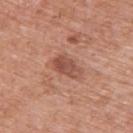Case summary:
- workup · total-body-photography surveillance lesion; no biopsy
- subject · male, aged 78–82
- anatomic site · the back
- automated lesion analysis · a lesion area of about 6 mm², a shape eccentricity near 0.8, and two-axis asymmetry of about 0.2; an automated nevus-likeness rating near 20 out of 100 and a detector confidence of about 100 out of 100 that the crop contains a lesion
- image source · ~15 mm tile from a whole-body skin photo
- lighting · white-light illumination
- diameter · ~3.5 mm (longest diameter)